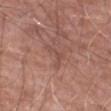• workup — catalogued during a skin exam; not biopsied
• illumination — white-light
• patient — male, aged around 60
• anatomic site — the right upper arm
• acquisition — 15 mm crop, total-body photography
• diameter — ≈3.5 mm